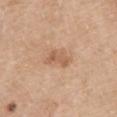workup: imaged on a skin check; not biopsied
automated lesion analysis: a lesion area of about 5 mm², a shape eccentricity near 0.85, and a symmetry-axis asymmetry near 0.4; a lesion color around L≈59 a*≈20 b*≈34 in CIELAB, a lesion–skin lightness drop of about 9, and a lesion-to-skin contrast of about 6.5 (normalized; higher = more distinct); a border-irregularity rating of about 4/10, a within-lesion color-variation index near 3/10, and radial color variation of about 1; a nevus-likeness score of about 5/100 and a lesion-detection confidence of about 100/100
anatomic site: the upper back
lesion diameter: about 3.5 mm
imaging modality: ~15 mm crop, total-body skin-cancer survey
illumination: white-light illumination
subject: female, aged approximately 60